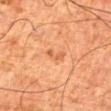– workup: no biopsy performed (imaged during a skin exam)
– body site: the leg
– image: ~15 mm crop, total-body skin-cancer survey
– subject: male, in their 80s